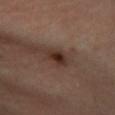{
  "biopsy_status": "not biopsied; imaged during a skin examination"
}